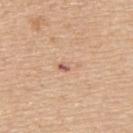{
  "lesion_size": {
    "long_diameter_mm_approx": 3.0
  },
  "site": "upper back",
  "patient": {
    "sex": "male",
    "age_approx": 65
  },
  "lighting": "white-light",
  "image": {
    "source": "total-body photography crop",
    "field_of_view_mm": 15
  }
}